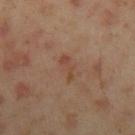Captured during whole-body skin photography for melanoma surveillance; the lesion was not biopsied. Cropped from a total-body skin-imaging series; the visible field is about 15 mm. From the left upper arm. A male patient about 45 years old.